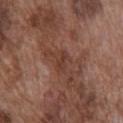Assessment: No biopsy was performed on this lesion — it was imaged during a full skin examination and was not determined to be concerning. Background: A male subject in their mid-70s. The lesion is located on the front of the torso. A region of skin cropped from a whole-body photographic capture, roughly 15 mm wide.